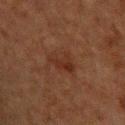biopsy status: catalogued during a skin exam; not biopsied
automated lesion analysis: an average lesion color of about L≈24 a*≈18 b*≈23 (CIELAB), roughly 5 lightness units darker than nearby skin, and a normalized border contrast of about 6; internal color variation of about 2.5 on a 0–10 scale
lesion diameter: ≈4 mm
imaging modality: 15 mm crop, total-body photography
patient: male, roughly 50 years of age
site: the upper back